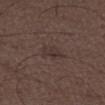biopsy status: catalogued during a skin exam; not biopsied
site: the right lower leg
subject: male, aged approximately 50
tile lighting: white-light illumination
image: total-body-photography crop, ~15 mm field of view
lesion diameter: ~2.5 mm (longest diameter)
automated lesion analysis: a lesion color around L≈32 a*≈13 b*≈17 in CIELAB, a lesion–skin lightness drop of about 5, and a normalized border contrast of about 5